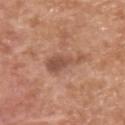notes=no biopsy performed (imaged during a skin exam); image source=total-body-photography crop, ~15 mm field of view; site=the chest; subject=male, in their mid- to late 60s; lesion diameter=about 5.5 mm.